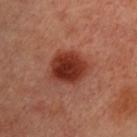biopsy status=total-body-photography surveillance lesion; no biopsy
image=total-body-photography crop, ~15 mm field of view
subject=female, aged around 55
site=the chest